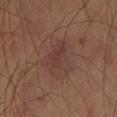- workup · imaged on a skin check; not biopsied
- TBP lesion metrics · an eccentricity of roughly 0.8 and two-axis asymmetry of about 0.4; a lesion color around L≈33 a*≈17 b*≈20 in CIELAB, about 5 CIELAB-L* units darker than the surrounding skin, and a lesion-to-skin contrast of about 5 (normalized; higher = more distinct)
- image · 15 mm crop, total-body photography
- tile lighting · cross-polarized
- site · the left thigh
- subject · male, in their mid-30s
- lesion size · about 5 mm